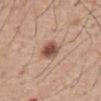workup: imaged on a skin check; not biopsied
image: 15 mm crop, total-body photography
illumination: white-light illumination
lesion diameter: about 3 mm
body site: the abdomen
patient: male, approximately 60 years of age